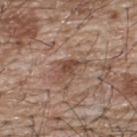Findings:
* workup — imaged on a skin check; not biopsied
* site — the upper back
* subject — male, aged 63 to 67
* acquisition — 15 mm crop, total-body photography